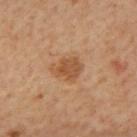The lesion was photographed on a routine skin check and not biopsied; there is no pathology result.